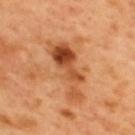The lesion was tiled from a total-body skin photograph and was not biopsied. Captured under cross-polarized illumination. The lesion is on the upper back. A 15 mm close-up tile from a total-body photography series done for melanoma screening. A male patient, approximately 50 years of age. Automated tile analysis of the lesion measured an area of roughly 14 mm², a shape eccentricity near 0.95, and two-axis asymmetry of about 0.55. The analysis additionally found a lesion color around L≈50 a*≈28 b*≈41 in CIELAB, a lesion–skin lightness drop of about 13, and a lesion-to-skin contrast of about 9 (normalized; higher = more distinct). And it measured a border-irregularity rating of about 8/10, a within-lesion color-variation index near 8.5/10, and radial color variation of about 2.5. And it measured a nevus-likeness score of about 20/100 and a detector confidence of about 100 out of 100 that the crop contains a lesion.